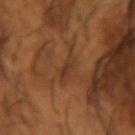biopsy status: catalogued during a skin exam; not biopsied
imaging modality: ~15 mm crop, total-body skin-cancer survey
patient: male, aged 63–67
site: the left forearm